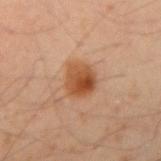workup: total-body-photography surveillance lesion; no biopsy.